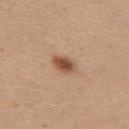<tbp_lesion>
  <biopsy_status>not biopsied; imaged during a skin examination</biopsy_status>
  <automated_metrics>
    <nevus_likeness_0_100>100</nevus_likeness_0_100>
    <lesion_detection_confidence_0_100>100</lesion_detection_confidence_0_100>
  </automated_metrics>
  <site>back</site>
  <lesion_size>
    <long_diameter_mm_approx>3.0</long_diameter_mm_approx>
  </lesion_size>
  <patient>
    <sex>female</sex>
    <age_approx>30</age_approx>
  </patient>
  <image>
    <source>total-body photography crop</source>
    <field_of_view_mm>15</field_of_view_mm>
  </image>
</tbp_lesion>